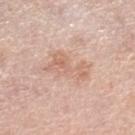Notes:
* notes · imaged on a skin check; not biopsied